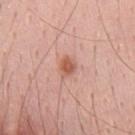biopsy status: no biopsy performed (imaged during a skin exam)
image source: ~15 mm tile from a whole-body skin photo
automated lesion analysis: an area of roughly 4 mm², an eccentricity of roughly 0.65, and two-axis asymmetry of about 0.2; a lesion–skin lightness drop of about 11 and a normalized border contrast of about 8
subject: male, approximately 55 years of age
illumination: white-light illumination
site: the chest
lesion size: ~2.5 mm (longest diameter)